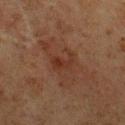Impression:
Imaged during a routine full-body skin examination; the lesion was not biopsied and no histopathology is available.
Context:
The lesion is on the chest. A male subject in their mid- to late 70s. This is a cross-polarized tile. A 15 mm close-up tile from a total-body photography series done for melanoma screening.